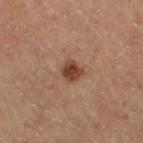Case summary:
– workup — total-body-photography surveillance lesion; no biopsy
– imaging modality — 15 mm crop, total-body photography
– automated lesion analysis — a nevus-likeness score of about 95/100 and a detector confidence of about 100 out of 100 that the crop contains a lesion
– subject — male, roughly 70 years of age
– lesion size — ≈2.5 mm
– anatomic site — the right thigh
– illumination — cross-polarized illumination